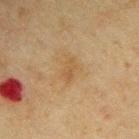{
  "biopsy_status": "not biopsied; imaged during a skin examination",
  "site": "right upper arm",
  "image": {
    "source": "total-body photography crop",
    "field_of_view_mm": 15
  },
  "patient": {
    "sex": "male",
    "age_approx": 70
  },
  "lesion_size": {
    "long_diameter_mm_approx": 3.0
  }
}